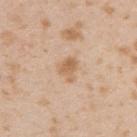Imaged during a routine full-body skin examination; the lesion was not biopsied and no histopathology is available. Automated image analysis of the tile measured an eccentricity of roughly 0.4 and two-axis asymmetry of about 0.3. It also reported a lesion color around L≈63 a*≈18 b*≈35 in CIELAB, about 9 CIELAB-L* units darker than the surrounding skin, and a normalized lesion–skin contrast near 7. The software also gave an automated nevus-likeness rating near 15 out of 100. About 2.5 mm across. The lesion is on the right upper arm. The tile uses white-light illumination. A male subject aged 23 to 27. A 15 mm close-up tile from a total-body photography series done for melanoma screening.